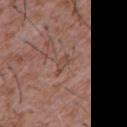– anatomic site: the chest
– imaging modality: ~15 mm tile from a whole-body skin photo
– size: ~2.5 mm (longest diameter)
– patient: male, in their mid- to late 40s